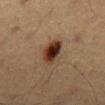- notes · no biopsy performed (imaged during a skin exam)
- imaging modality · ~15 mm crop, total-body skin-cancer survey
- patient · male, aged around 60
- anatomic site · the mid back
- image-analysis metrics · a mean CIELAB color near L≈26 a*≈15 b*≈22 and roughly 13 lightness units darker than nearby skin; a border-irregularity rating of about 2/10, internal color variation of about 7 on a 0–10 scale, and radial color variation of about 2